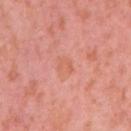| feature | finding |
|---|---|
| follow-up | imaged on a skin check; not biopsied |